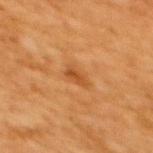| field | value |
|---|---|
| notes | total-body-photography surveillance lesion; no biopsy |
| size | ~2.5 mm (longest diameter) |
| anatomic site | the upper back |
| imaging modality | ~15 mm crop, total-body skin-cancer survey |
| image-analysis metrics | an area of roughly 3 mm² and a shape eccentricity near 0.85; a border-irregularity index near 3/10 and a color-variation rating of about 2/10; an automated nevus-likeness rating near 5 out of 100 |
| tile lighting | cross-polarized |
| subject | female, aged 53 to 57 |